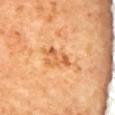follow-up — imaged on a skin check; not biopsied
image — ~15 mm tile from a whole-body skin photo
tile lighting — cross-polarized
location — the upper back
diameter — ~4 mm (longest diameter)
subject — female
image-analysis metrics — a lesion area of about 5 mm², an outline eccentricity of about 0.9 (0 = round, 1 = elongated), and a symmetry-axis asymmetry near 0.5; a border-irregularity rating of about 7/10, internal color variation of about 0.5 on a 0–10 scale, and radial color variation of about 0; a classifier nevus-likeness of about 0/100 and lesion-presence confidence of about 100/100